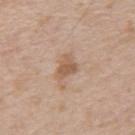Q: Is there a histopathology result?
A: no biopsy performed (imaged during a skin exam)